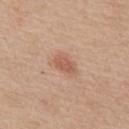Part of a total-body skin-imaging series; this lesion was reviewed on a skin check and was not flagged for biopsy. A male patient, aged around 60. Imaged with white-light lighting. On the upper back. Longest diameter approximately 2.5 mm. A roughly 15 mm field-of-view crop from a total-body skin photograph.